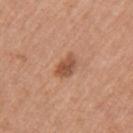This lesion was catalogued during total-body skin photography and was not selected for biopsy.
The lesion-visualizer software estimated a lesion area of about 5.5 mm², an eccentricity of roughly 0.75, and two-axis asymmetry of about 0.3. It also reported a nevus-likeness score of about 75/100 and a lesion-detection confidence of about 100/100.
The lesion is located on the arm.
A female subject, approximately 65 years of age.
Cropped from a whole-body photographic skin survey; the tile spans about 15 mm.
This is a white-light tile.